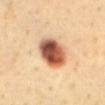A male patient, approximately 40 years of age. Located on the mid back. Cropped from a total-body skin-imaging series; the visible field is about 15 mm. Histopathology of the biopsied lesion showed a borderline lesion of uncertain malignant potential: atypical melanocytic neoplasm.